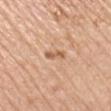Impression:
The lesion was photographed on a routine skin check and not biopsied; there is no pathology result.
Clinical summary:
The lesion-visualizer software estimated a lesion area of about 2.5 mm², an outline eccentricity of about 0.95 (0 = round, 1 = elongated), and a shape-asymmetry score of about 0.4 (0 = symmetric). And it measured about 11 CIELAB-L* units darker than the surrounding skin and a normalized border contrast of about 7. And it measured a border-irregularity index near 4.5/10 and peripheral color asymmetry of about 0. Located on the left upper arm. A male subject in their mid-70s. The tile uses white-light illumination. Cropped from a total-body skin-imaging series; the visible field is about 15 mm. The lesion's longest dimension is about 3 mm.